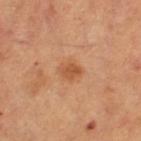Assessment: Part of a total-body skin-imaging series; this lesion was reviewed on a skin check and was not flagged for biopsy. Context: Captured under cross-polarized illumination. A female subject, aged 58–62. On the left thigh. A close-up tile cropped from a whole-body skin photograph, about 15 mm across.